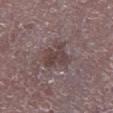Impression:
Imaged during a routine full-body skin examination; the lesion was not biopsied and no histopathology is available.
Context:
An algorithmic analysis of the crop reported an outline eccentricity of about 0.55 (0 = round, 1 = elongated) and a symmetry-axis asymmetry near 0.35. It also reported a lesion color around L≈42 a*≈15 b*≈16 in CIELAB and a normalized border contrast of about 7. And it measured a border-irregularity rating of about 4.5/10, a within-lesion color-variation index near 4/10, and a peripheral color-asymmetry measure near 1.5. And it measured a nevus-likeness score of about 0/100 and a lesion-detection confidence of about 70/100. Imaged with white-light lighting. A male patient aged 78 to 82. The lesion is located on the right lower leg. This image is a 15 mm lesion crop taken from a total-body photograph.